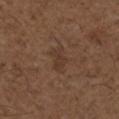<lesion>
  <biopsy_status>not biopsied; imaged during a skin examination</biopsy_status>
  <site>left upper arm</site>
  <lesion_size>
    <long_diameter_mm_approx>3.0</long_diameter_mm_approx>
  </lesion_size>
  <image>
    <source>total-body photography crop</source>
    <field_of_view_mm>15</field_of_view_mm>
  </image>
  <patient>
    <sex>male</sex>
    <age_approx>50</age_approx>
  </patient>
</lesion>